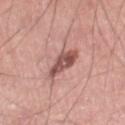biopsy status: no biopsy performed (imaged during a skin exam)
size: ≈4 mm
automated lesion analysis: border irregularity of about 3 on a 0–10 scale, a within-lesion color-variation index near 4.5/10, and peripheral color asymmetry of about 1.5
patient: male, aged approximately 70
anatomic site: the right thigh
acquisition: 15 mm crop, total-body photography